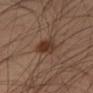Recorded during total-body skin imaging; not selected for excision or biopsy. From the right thigh. Automated tile analysis of the lesion measured border irregularity of about 3 on a 0–10 scale and a peripheral color-asymmetry measure near 1. And it measured lesion-presence confidence of about 100/100. A male subject, aged 53 to 57. Cropped from a total-body skin-imaging series; the visible field is about 15 mm. Imaged with cross-polarized lighting. Longest diameter approximately 4 mm.